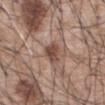Assessment:
No biopsy was performed on this lesion — it was imaged during a full skin examination and was not determined to be concerning.
Context:
This image is a 15 mm lesion crop taken from a total-body photograph. The lesion is on the abdomen. A male patient approximately 60 years of age. This is a white-light tile.